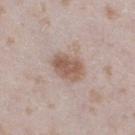follow-up = no biopsy performed (imaged during a skin exam)
image = ~15 mm tile from a whole-body skin photo
body site = the right thigh
image-analysis metrics = about 11 CIELAB-L* units darker than the surrounding skin and a normalized lesion–skin contrast near 8; border irregularity of about 1.5 on a 0–10 scale, a color-variation rating of about 3/10, and a peripheral color-asymmetry measure near 1; a nevus-likeness score of about 85/100 and a lesion-detection confidence of about 100/100
tile lighting = white-light
subject = female, roughly 25 years of age